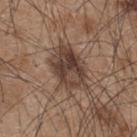The lesion was photographed on a routine skin check and not biopsied; there is no pathology result. Imaged with white-light lighting. Located on the upper back. Approximately 6 mm at its widest. A male subject, roughly 45 years of age. A close-up tile cropped from a whole-body skin photograph, about 15 mm across.